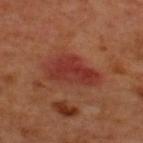notes: catalogued during a skin exam; not biopsied
image: ~15 mm crop, total-body skin-cancer survey
site: the back
diameter: ~6 mm (longest diameter)
tile lighting: cross-polarized
patient: male, in their 50s